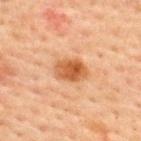notes: no biopsy performed (imaged during a skin exam)
subject: male, approximately 60 years of age
lighting: cross-polarized illumination
diameter: about 3.5 mm
automated metrics: a footprint of about 8 mm², an eccentricity of roughly 0.7, and a shape-asymmetry score of about 0.2 (0 = symmetric)
image: ~15 mm crop, total-body skin-cancer survey
site: the upper back